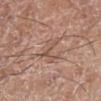The lesion was photographed on a routine skin check and not biopsied; there is no pathology result. A male patient aged around 60. The lesion is on the left lower leg. A roughly 15 mm field-of-view crop from a total-body skin photograph. The lesion's longest dimension is about 4 mm. Captured under white-light illumination.